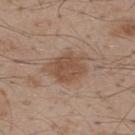{"biopsy_status": "not biopsied; imaged during a skin examination", "image": {"source": "total-body photography crop", "field_of_view_mm": 15}, "lighting": "white-light", "site": "upper back", "patient": {"sex": "male", "age_approx": 50}, "lesion_size": {"long_diameter_mm_approx": 5.5}}